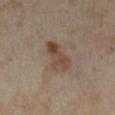{"biopsy_status": "not biopsied; imaged during a skin examination", "patient": {"sex": "female", "age_approx": 35}, "image": {"source": "total-body photography crop", "field_of_view_mm": 15}, "site": "right lower leg"}